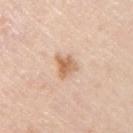biopsy status: imaged on a skin check; not biopsied
site: the right upper arm
image: ~15 mm crop, total-body skin-cancer survey
subject: male, roughly 45 years of age